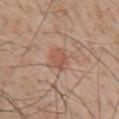Case summary:
* follow-up · catalogued during a skin exam; not biopsied
* acquisition · total-body-photography crop, ~15 mm field of view
* body site · the chest
* patient · male, aged 58 to 62